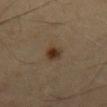Assessment:
No biopsy was performed on this lesion — it was imaged during a full skin examination and was not determined to be concerning.
Acquisition and patient details:
The lesion is located on the mid back. The patient is a male roughly 40 years of age. A 15 mm close-up tile from a total-body photography series done for melanoma screening. Automated tile analysis of the lesion measured border irregularity of about 2 on a 0–10 scale, a color-variation rating of about 4.5/10, and peripheral color asymmetry of about 1.5. Measured at roughly 2.5 mm in maximum diameter.